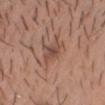Acquisition and patient details:
From the chest. The patient is a male about 20 years old. Imaged with white-light lighting. About 3 mm across. Automated image analysis of the tile measured an area of roughly 4 mm², an outline eccentricity of about 0.8 (0 = round, 1 = elongated), and a shape-asymmetry score of about 0.25 (0 = symmetric). The software also gave an average lesion color of about L≈48 a*≈20 b*≈26 (CIELAB), roughly 8 lightness units darker than nearby skin, and a normalized lesion–skin contrast near 6.5. The analysis additionally found a border-irregularity index near 3/10, a within-lesion color-variation index near 3/10, and a peripheral color-asymmetry measure near 1. Cropped from a total-body skin-imaging series; the visible field is about 15 mm.